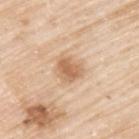automated metrics: an area of roughly 7.5 mm², an outline eccentricity of about 0.5 (0 = round, 1 = elongated), and a shape-asymmetry score of about 0.25 (0 = symmetric); a border-irregularity rating of about 2/10, a color-variation rating of about 3.5/10, and peripheral color asymmetry of about 1; lesion-presence confidence of about 100/100
image: ~15 mm crop, total-body skin-cancer survey
illumination: white-light
size: about 3 mm
subject: male, aged around 80
site: the upper back